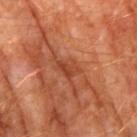Imaged with cross-polarized lighting.
A male subject in their 60s.
A 15 mm close-up extracted from a 3D total-body photography capture.
The lesion's longest dimension is about 2.5 mm.
From the arm.